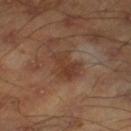Findings:
• location: the right lower leg
• subject: male, aged 43–47
• imaging modality: total-body-photography crop, ~15 mm field of view
• automated metrics: a lesion area of about 7.5 mm² and two-axis asymmetry of about 0.35; an automated nevus-likeness rating near 0 out of 100 and a detector confidence of about 100 out of 100 that the crop contains a lesion